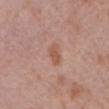follow-up: imaged on a skin check; not biopsied | subject: female, in their mid- to late 70s | tile lighting: white-light | diameter: ~3 mm (longest diameter) | body site: the front of the torso | image source: ~15 mm tile from a whole-body skin photo.